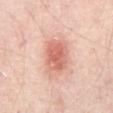• notes: catalogued during a skin exam; not biopsied
• acquisition: ~15 mm tile from a whole-body skin photo
• diameter: ~4 mm (longest diameter)
• patient: in their mid-50s
• tile lighting: cross-polarized
• location: the abdomen
• automated metrics: two-axis asymmetry of about 0.15; a mean CIELAB color near L≈63 a*≈26 b*≈28, roughly 12 lightness units darker than nearby skin, and a normalized lesion–skin contrast near 7